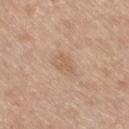workup=imaged on a skin check; not biopsied | acquisition=~15 mm tile from a whole-body skin photo | body site=the right thigh | subject=male, roughly 70 years of age.